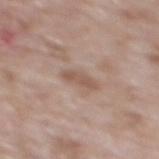The lesion was photographed on a routine skin check and not biopsied; there is no pathology result.
Automated image analysis of the tile measured an area of roughly 4 mm² and two-axis asymmetry of about 0.3. It also reported border irregularity of about 3 on a 0–10 scale, internal color variation of about 1.5 on a 0–10 scale, and peripheral color asymmetry of about 0.5. And it measured a classifier nevus-likeness of about 0/100 and lesion-presence confidence of about 100/100.
A 15 mm close-up tile from a total-body photography series done for melanoma screening.
The subject is a male aged 58 to 62.
Captured under white-light illumination.
The lesion is on the mid back.